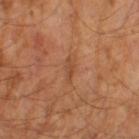Recorded during total-body skin imaging; not selected for excision or biopsy. Cropped from a whole-body photographic skin survey; the tile spans about 15 mm. About 2.5 mm across. Captured under cross-polarized illumination. The patient is a male aged around 65.